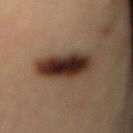Notes:
- notes · no biopsy performed (imaged during a skin exam)
- patient · male, in their mid- to late 60s
- illumination · cross-polarized
- body site · the back
- imaging modality · 15 mm crop, total-body photography
- TBP lesion metrics · a shape eccentricity near 0.85 and a symmetry-axis asymmetry near 0.2; a border-irregularity index near 2.5/10, a color-variation rating of about 8/10, and radial color variation of about 2.5
- lesion size · ~6.5 mm (longest diameter)